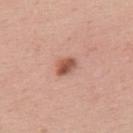Recorded during total-body skin imaging; not selected for excision or biopsy. The recorded lesion diameter is about 2.5 mm. A lesion tile, about 15 mm wide, cut from a 3D total-body photograph. The lesion is on the upper back. Captured under white-light illumination. A female subject about 35 years old.